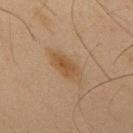biopsy_status: not biopsied; imaged during a skin examination
site: upper back
lesion_size:
  long_diameter_mm_approx: 5.0
automated_metrics:
  area_mm2_approx: 8.0
  eccentricity: 0.9
  cielab_L: 41
  cielab_a: 14
  cielab_b: 29
  vs_skin_darker_L: 7.0
  vs_skin_contrast_norm: 7.0
  lesion_detection_confidence_0_100: 100
patient:
  sex: male
  age_approx: 65
image:
  source: total-body photography crop
  field_of_view_mm: 15